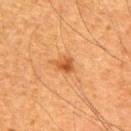biopsy status: catalogued during a skin exam; not biopsied | image source: ~15 mm tile from a whole-body skin photo | body site: the upper back | lesion diameter: ~2.5 mm (longest diameter) | patient: male, aged approximately 65 | image-analysis metrics: a mean CIELAB color near L≈46 a*≈25 b*≈38, roughly 10 lightness units darker than nearby skin, and a normalized border contrast of about 7.5; an automated nevus-likeness rating near 75 out of 100 and a lesion-detection confidence of about 100/100.